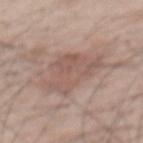Clinical impression: The lesion was tiled from a total-body skin photograph and was not biopsied. Context: Cropped from a whole-body photographic skin survey; the tile spans about 15 mm. The lesion is located on the mid back. A male subject, roughly 55 years of age. The lesion-visualizer software estimated a footprint of about 17 mm² and an outline eccentricity of about 0.7 (0 = round, 1 = elongated). It also reported a border-irregularity rating of about 4.5/10 and internal color variation of about 3.5 on a 0–10 scale. And it measured an automated nevus-likeness rating near 15 out of 100 and a lesion-detection confidence of about 100/100. Imaged with white-light lighting. The lesion's longest dimension is about 6 mm.